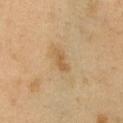Clinical impression:
No biopsy was performed on this lesion — it was imaged during a full skin examination and was not determined to be concerning.
Context:
A male subject, aged 48 to 52. The lesion is on the right upper arm. A lesion tile, about 15 mm wide, cut from a 3D total-body photograph.